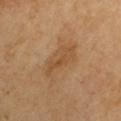| feature | finding |
|---|---|
| diameter | ≈5.5 mm |
| image | ~15 mm crop, total-body skin-cancer survey |
| site | the left arm |
| subject | male, aged 63–67 |